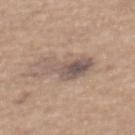The lesion was photographed on a routine skin check and not biopsied; there is no pathology result.
Cropped from a whole-body photographic skin survey; the tile spans about 15 mm.
The lesion is located on the mid back.
Captured under white-light illumination.
A male subject in their mid- to late 60s.
The recorded lesion diameter is about 5.5 mm.